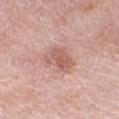Q: Was a biopsy performed?
A: catalogued during a skin exam; not biopsied
Q: What kind of image is this?
A: total-body-photography crop, ~15 mm field of view
Q: How was the tile lit?
A: white-light
Q: What did automated image analysis measure?
A: a footprint of about 7 mm², a shape eccentricity near 0.75, and a symmetry-axis asymmetry near 0.25; a classifier nevus-likeness of about 35/100
Q: Who is the patient?
A: female, approximately 70 years of age
Q: Lesion location?
A: the leg
Q: Lesion size?
A: ~3.5 mm (longest diameter)